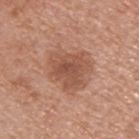{
  "biopsy_status": "not biopsied; imaged during a skin examination",
  "site": "upper back",
  "lesion_size": {
    "long_diameter_mm_approx": 4.5
  },
  "lighting": "white-light",
  "patient": {
    "sex": "male",
    "age_approx": 55
  },
  "image": {
    "source": "total-body photography crop",
    "field_of_view_mm": 15
  }
}